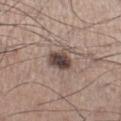Cropped from a whole-body photographic skin survey; the tile spans about 15 mm.
Imaged with white-light lighting.
An algorithmic analysis of the crop reported an area of roughly 6 mm². The software also gave border irregularity of about 1.5 on a 0–10 scale, internal color variation of about 4.5 on a 0–10 scale, and peripheral color asymmetry of about 1.5. It also reported a nevus-likeness score of about 85/100.
The lesion is on the right lower leg.
A male subject aged 58 to 62.